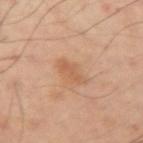  biopsy_status: not biopsied; imaged during a skin examination
  lesion_size:
    long_diameter_mm_approx: 3.5
  lighting: cross-polarized
  image:
    source: total-body photography crop
    field_of_view_mm: 15
  site: left arm
  patient:
    sex: male
    age_approx: 50